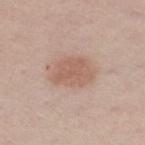follow-up: catalogued during a skin exam; not biopsied | imaging modality: ~15 mm crop, total-body skin-cancer survey | body site: the leg | automated metrics: a lesion color around L≈59 a*≈20 b*≈27 in CIELAB, a lesion–skin lightness drop of about 9, and a normalized lesion–skin contrast near 6; a border-irregularity rating of about 2.5/10 and a peripheral color-asymmetry measure near 0.5; a classifier nevus-likeness of about 95/100 | illumination: white-light | subject: male, roughly 60 years of age | lesion size: ≈5 mm.